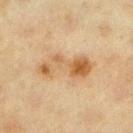biopsy status — total-body-photography surveillance lesion; no biopsy | patient — female, aged around 40 | location — the left lower leg | acquisition — 15 mm crop, total-body photography.